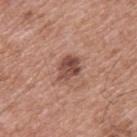workup: imaged on a skin check; not biopsied
automated metrics: a detector confidence of about 100 out of 100 that the crop contains a lesion
size: ~3.5 mm (longest diameter)
subject: male, in their mid-50s
image: ~15 mm tile from a whole-body skin photo
site: the upper back
lighting: white-light